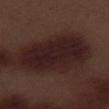Captured during whole-body skin photography for melanoma surveillance; the lesion was not biopsied. The lesion is located on the right thigh. A male subject, aged 68 to 72. Cropped from a total-body skin-imaging series; the visible field is about 15 mm. An algorithmic analysis of the crop reported a lesion area of about 33 mm², a shape eccentricity near 0.9, and a shape-asymmetry score of about 0.15 (0 = symmetric). It also reported a lesion color around L≈20 a*≈17 b*≈15 in CIELAB and a normalized border contrast of about 12. The analysis additionally found border irregularity of about 2.5 on a 0–10 scale, a within-lesion color-variation index near 3/10, and radial color variation of about 1. Captured under white-light illumination. Approximately 10 mm at its widest.